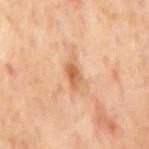{
  "biopsy_status": "not biopsied; imaged during a skin examination",
  "lighting": "cross-polarized",
  "site": "mid back",
  "automated_metrics": {
    "area_mm2_approx": 5.5,
    "eccentricity": 0.9,
    "shape_asymmetry": 0.3,
    "cielab_L": 61,
    "cielab_a": 22,
    "cielab_b": 38,
    "vs_skin_darker_L": 10.0,
    "vs_skin_contrast_norm": 7.0
  },
  "patient": {
    "sex": "male",
    "age_approx": 65
  },
  "lesion_size": {
    "long_diameter_mm_approx": 4.0
  },
  "image": {
    "source": "total-body photography crop",
    "field_of_view_mm": 15
  }
}